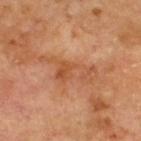biopsy status: catalogued during a skin exam; not biopsied
image: total-body-photography crop, ~15 mm field of view
anatomic site: the upper back
patient: male, aged 68–72
tile lighting: cross-polarized